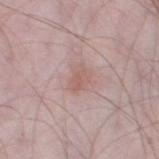| key | value |
|---|---|
| notes | total-body-photography surveillance lesion; no biopsy |
| subject | male, in their mid- to late 50s |
| imaging modality | ~15 mm crop, total-body skin-cancer survey |
| site | the left thigh |